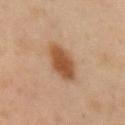– anatomic site · the right upper arm
– automated lesion analysis · a footprint of about 11 mm²; an average lesion color of about L≈41 a*≈17 b*≈29 (CIELAB), about 10 CIELAB-L* units darker than the surrounding skin, and a normalized lesion–skin contrast near 9; a nevus-likeness score of about 100/100 and a detector confidence of about 100 out of 100 that the crop contains a lesion
– image · total-body-photography crop, ~15 mm field of view
– size · ~5 mm (longest diameter)
– subject · male, about 55 years old
– tile lighting · cross-polarized